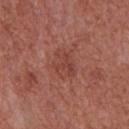Imaged during a routine full-body skin examination; the lesion was not biopsied and no histopathology is available.
The lesion-visualizer software estimated a lesion color around L≈43 a*≈26 b*≈27 in CIELAB, about 6 CIELAB-L* units darker than the surrounding skin, and a normalized border contrast of about 5. And it measured a classifier nevus-likeness of about 5/100.
The lesion is on the chest.
A close-up tile cropped from a whole-body skin photograph, about 15 mm across.
Longest diameter approximately 3.5 mm.
A male subject, roughly 65 years of age.
The tile uses white-light illumination.